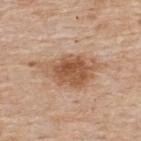<tbp_lesion>
  <biopsy_status>not biopsied; imaged during a skin examination</biopsy_status>
  <image>
    <source>total-body photography crop</source>
    <field_of_view_mm>15</field_of_view_mm>
  </image>
  <lesion_size>
    <long_diameter_mm_approx>9.0</long_diameter_mm_approx>
  </lesion_size>
  <site>upper back</site>
  <patient>
    <sex>male</sex>
    <age_approx>55</age_approx>
  </patient>
</tbp_lesion>